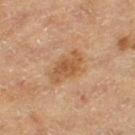The lesion is located on the left thigh.
About 4.5 mm across.
A lesion tile, about 15 mm wide, cut from a 3D total-body photograph.
A female patient aged approximately 55.
Captured under cross-polarized illumination.